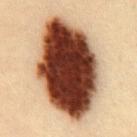follow-up=catalogued during a skin exam; not biopsied | lighting=cross-polarized illumination | site=the chest | patient=male, aged 33–37 | image source=total-body-photography crop, ~15 mm field of view | lesion size=about 12 mm.